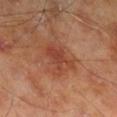biopsy status — imaged on a skin check; not biopsied | subject — male, approximately 70 years of age | diameter — about 4.5 mm | location — the right lower leg | image — total-body-photography crop, ~15 mm field of view | tile lighting — cross-polarized illumination.